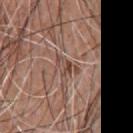| field | value |
|---|---|
| notes | total-body-photography surveillance lesion; no biopsy |
| diameter | ~3 mm (longest diameter) |
| patient | male, roughly 55 years of age |
| image | ~15 mm crop, total-body skin-cancer survey |
| location | the front of the torso |
| illumination | white-light illumination |